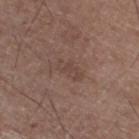Part of a total-body skin-imaging series; this lesion was reviewed on a skin check and was not flagged for biopsy.